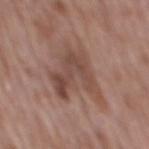Assessment:
The lesion was photographed on a routine skin check and not biopsied; there is no pathology result.
Context:
Cropped from a whole-body photographic skin survey; the tile spans about 15 mm. Located on the back. A male subject aged around 70.